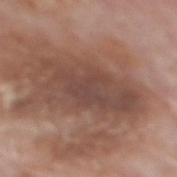Recorded during total-body skin imaging; not selected for excision or biopsy.
From the mid back.
The patient is a male in their mid-70s.
This image is a 15 mm lesion crop taken from a total-body photograph.
Automated image analysis of the tile measured a footprint of about 27 mm² and a shape-asymmetry score of about 0.25 (0 = symmetric). It also reported an average lesion color of about L≈45 a*≈19 b*≈24 (CIELAB) and a normalized border contrast of about 7.5. It also reported an automated nevus-likeness rating near 0 out of 100 and a detector confidence of about 95 out of 100 that the crop contains a lesion.
The tile uses white-light illumination.
Longest diameter approximately 9 mm.